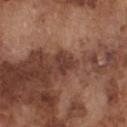Clinical impression:
Part of a total-body skin-imaging series; this lesion was reviewed on a skin check and was not flagged for biopsy.
Clinical summary:
This is a white-light tile. The lesion's longest dimension is about 2.5 mm. Cropped from a whole-body photographic skin survey; the tile spans about 15 mm. The lesion is located on the chest. A male patient aged 73–77.